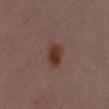The lesion was photographed on a routine skin check and not biopsied; there is no pathology result. The tile uses cross-polarized illumination. A male patient, in their 30s. A 15 mm close-up extracted from a 3D total-body photography capture. The lesion's longest dimension is about 4 mm. The lesion is located on the left upper arm.